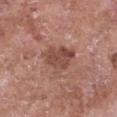Q: What is the anatomic site?
A: the chest
Q: How was this image acquired?
A: ~15 mm crop, total-body skin-cancer survey
Q: What are the patient's age and sex?
A: female, aged 73–77